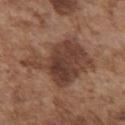A male patient roughly 75 years of age. Imaged with white-light lighting. Located on the front of the torso. Cropped from a whole-body photographic skin survey; the tile spans about 15 mm. Approximately 7.5 mm at its widest.